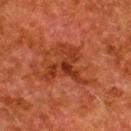No biopsy was performed on this lesion — it was imaged during a full skin examination and was not determined to be concerning. The lesion is on the upper back. A roughly 15 mm field-of-view crop from a total-body skin photograph. Longest diameter approximately 5 mm. The tile uses cross-polarized illumination. An algorithmic analysis of the crop reported a lesion color around L≈31 a*≈27 b*≈32 in CIELAB and a normalized border contrast of about 7. The analysis additionally found a border-irregularity index near 9.5/10. The software also gave a nevus-likeness score of about 0/100. A female subject, in their 50s.